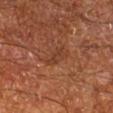biopsy status = no biopsy performed (imaged during a skin exam); diameter = ~3 mm (longest diameter); subject = male, roughly 65 years of age; acquisition = total-body-photography crop, ~15 mm field of view; anatomic site = the right lower leg.